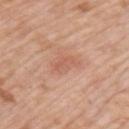A 15 mm close-up tile from a total-body photography series done for melanoma screening. Captured under white-light illumination. A male patient aged 73 to 77. The lesion-visualizer software estimated a border-irregularity index near 4/10, a within-lesion color-variation index near 1.5/10, and a peripheral color-asymmetry measure near 0.5. Approximately 3 mm at its widest. Located on the left upper arm.